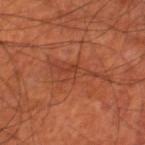Image and clinical context: A male patient roughly 70 years of age. Located on the right thigh. A roughly 15 mm field-of-view crop from a total-body skin photograph.